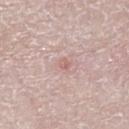The lesion was photographed on a routine skin check and not biopsied; there is no pathology result. This image is a 15 mm lesion crop taken from a total-body photograph. Longest diameter approximately 1.5 mm. The lesion is located on the right lower leg. Imaged with white-light lighting. The subject is a male aged 78 to 82.